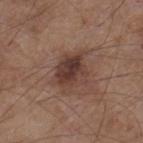The lesion was photographed on a routine skin check and not biopsied; there is no pathology result. The patient is a male aged 53–57. This image is a 15 mm lesion crop taken from a total-body photograph. Imaged with white-light lighting. The lesion is on the right lower leg. The lesion-visualizer software estimated an area of roughly 12 mm², an outline eccentricity of about 0.6 (0 = round, 1 = elongated), and two-axis asymmetry of about 0.25. The analysis additionally found a mean CIELAB color near L≈39 a*≈18 b*≈23 and roughly 10 lightness units darker than nearby skin. The analysis additionally found a border-irregularity rating of about 3/10, a within-lesion color-variation index near 6/10, and radial color variation of about 2. The software also gave a nevus-likeness score of about 40/100 and a detector confidence of about 100 out of 100 that the crop contains a lesion.